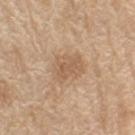{
  "biopsy_status": "not biopsied; imaged during a skin examination",
  "automated_metrics": {
    "area_mm2_approx": 9.0,
    "eccentricity": 0.6,
    "shape_asymmetry": 0.3,
    "cielab_L": 59,
    "cielab_a": 17,
    "cielab_b": 33,
    "vs_skin_darker_L": 8.0,
    "vs_skin_contrast_norm": 5.5,
    "color_variation_0_10": 2.0,
    "peripheral_color_asymmetry": 0.5
  },
  "site": "right upper arm",
  "lighting": "white-light",
  "patient": {
    "sex": "male",
    "age_approx": 70
  },
  "image": {
    "source": "total-body photography crop",
    "field_of_view_mm": 15
  },
  "lesion_size": {
    "long_diameter_mm_approx": 4.0
  }
}